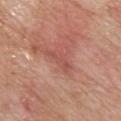Imaged during a routine full-body skin examination; the lesion was not biopsied and no histopathology is available. A 15 mm crop from a total-body photograph taken for skin-cancer surveillance. The subject is a female aged approximately 70. Imaged with white-light lighting. The lesion is located on the chest.